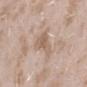| feature | finding |
|---|---|
| biopsy status | no biopsy performed (imaged during a skin exam) |
| acquisition | ~15 mm crop, total-body skin-cancer survey |
| site | the left forearm |
| tile lighting | white-light illumination |
| image-analysis metrics | an area of roughly 6 mm², an outline eccentricity of about 0.95 (0 = round, 1 = elongated), and two-axis asymmetry of about 0.55; a mean CIELAB color near L≈60 a*≈16 b*≈28, roughly 9 lightness units darker than nearby skin, and a lesion-to-skin contrast of about 6.5 (normalized; higher = more distinct); a nevus-likeness score of about 0/100 and a detector confidence of about 100 out of 100 that the crop contains a lesion |
| size | ~5 mm (longest diameter) |
| patient | female, roughly 25 years of age |